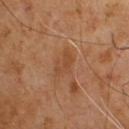The lesion was photographed on a routine skin check and not biopsied; there is no pathology result. A male patient, roughly 65 years of age. Imaged with cross-polarized lighting. About 3.5 mm across. The total-body-photography lesion software estimated a lesion area of about 6 mm², an outline eccentricity of about 0.8 (0 = round, 1 = elongated), and two-axis asymmetry of about 0.3. And it measured a border-irregularity rating of about 3.5/10, a within-lesion color-variation index near 2.5/10, and peripheral color asymmetry of about 1. The analysis additionally found an automated nevus-likeness rating near 0 out of 100 and lesion-presence confidence of about 100/100. A 15 mm close-up tile from a total-body photography series done for melanoma screening.